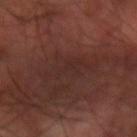Notes:
* workup: catalogued during a skin exam; not biopsied
* automated lesion analysis: an area of roughly 11 mm² and a symmetry-axis asymmetry near 0.55; an average lesion color of about L≈26 a*≈19 b*≈20 (CIELAB) and a normalized lesion–skin contrast near 4.5; internal color variation of about 2 on a 0–10 scale and a peripheral color-asymmetry measure near 0.5
* acquisition: ~15 mm tile from a whole-body skin photo
* diameter: ~5.5 mm (longest diameter)
* tile lighting: cross-polarized illumination
* patient: male, approximately 60 years of age
* location: the right arm